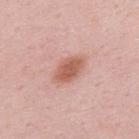Case summary:
• follow-up: total-body-photography surveillance lesion; no biopsy
• diameter: about 4 mm
• image: ~15 mm crop, total-body skin-cancer survey
• body site: the upper back
• illumination: white-light
• subject: male, roughly 50 years of age